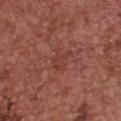{"biopsy_status": "not biopsied; imaged during a skin examination", "site": "upper back", "image": {"source": "total-body photography crop", "field_of_view_mm": 15}, "patient": {"sex": "female", "age_approx": 65}, "lesion_size": {"long_diameter_mm_approx": 3.0}, "lighting": "white-light", "automated_metrics": {"eccentricity": 0.6, "shape_asymmetry": 0.35, "border_irregularity_0_10": 4.5, "color_variation_0_10": 1.5, "nevus_likeness_0_100": 0}}